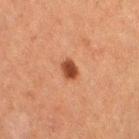Imaged during a routine full-body skin examination; the lesion was not biopsied and no histopathology is available. The recorded lesion diameter is about 2.5 mm. A female subject, approximately 50 years of age. Imaged with cross-polarized lighting. A 15 mm close-up extracted from a 3D total-body photography capture. From the leg. The total-body-photography lesion software estimated a lesion area of about 4 mm², an eccentricity of roughly 0.65, and a symmetry-axis asymmetry near 0.25. It also reported about 13 CIELAB-L* units darker than the surrounding skin and a lesion-to-skin contrast of about 10.5 (normalized; higher = more distinct).